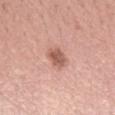image:
  source: total-body photography crop
  field_of_view_mm: 15
site: right forearm
patient:
  sex: female
  age_approx: 45